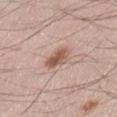follow-up: total-body-photography surveillance lesion; no biopsy
anatomic site: the left thigh
subject: male, aged approximately 45
lesion size: ~3 mm (longest diameter)
illumination: white-light
image: total-body-photography crop, ~15 mm field of view
TBP lesion metrics: a lesion color around L≈56 a*≈20 b*≈28 in CIELAB, about 12 CIELAB-L* units darker than the surrounding skin, and a normalized border contrast of about 8.5